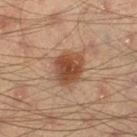notes = total-body-photography surveillance lesion; no biopsy | illumination = cross-polarized illumination | anatomic site = the left thigh | subject = male, aged around 45 | image source = ~15 mm tile from a whole-body skin photo.